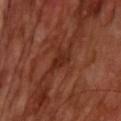Part of a total-body skin-imaging series; this lesion was reviewed on a skin check and was not flagged for biopsy.
A male patient, in their mid- to late 60s.
This image is a 15 mm lesion crop taken from a total-body photograph.